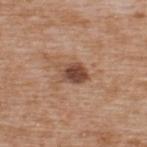Recorded during total-body skin imaging; not selected for excision or biopsy. Located on the upper back. An algorithmic analysis of the crop reported a lesion–skin lightness drop of about 13 and a lesion-to-skin contrast of about 9.5 (normalized; higher = more distinct). The analysis additionally found a border-irregularity index near 2.5/10, a within-lesion color-variation index near 5/10, and peripheral color asymmetry of about 2. And it measured a classifier nevus-likeness of about 55/100 and lesion-presence confidence of about 100/100. Cropped from a whole-body photographic skin survey; the tile spans about 15 mm. Measured at roughly 3.5 mm in maximum diameter. The tile uses white-light illumination. A male subject, aged around 65.